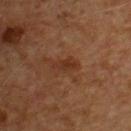Clinical impression: The lesion was tiled from a total-body skin photograph and was not biopsied. Clinical summary: A male patient about 55 years old. The lesion's longest dimension is about 3.5 mm. A roughly 15 mm field-of-view crop from a total-body skin photograph. An algorithmic analysis of the crop reported a lesion area of about 5 mm² and a shape eccentricity near 0.85. The analysis additionally found a classifier nevus-likeness of about 10/100 and a detector confidence of about 100 out of 100 that the crop contains a lesion. Located on the chest. The tile uses cross-polarized illumination.